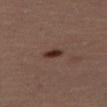An algorithmic analysis of the crop reported a lesion area of about 3.5 mm² and two-axis asymmetry of about 0.2. It also reported a border-irregularity index near 2/10, internal color variation of about 3 on a 0–10 scale, and radial color variation of about 1. The software also gave a nevus-likeness score of about 100/100 and a lesion-detection confidence of about 100/100. The lesion is located on the right thigh. A female subject, in their mid- to late 40s. A roughly 15 mm field-of-view crop from a total-body skin photograph. Imaged with cross-polarized lighting.